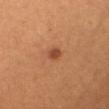Findings:
– workup · catalogued during a skin exam; not biopsied
– subject · female, aged around 40
– diameter · ~2 mm (longest diameter)
– automated lesion analysis · a normalized lesion–skin contrast near 7.5
– acquisition · 15 mm crop, total-body photography
– tile lighting · cross-polarized
– body site · the abdomen